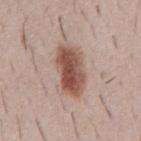Q: Was a biopsy performed?
A: total-body-photography surveillance lesion; no biopsy
Q: What is the imaging modality?
A: ~15 mm crop, total-body skin-cancer survey
Q: Who is the patient?
A: male, about 50 years old
Q: Illumination type?
A: white-light illumination
Q: What is the lesion's diameter?
A: ~6 mm (longest diameter)
Q: Automated lesion metrics?
A: a lesion area of about 15 mm², a shape eccentricity near 0.85, and two-axis asymmetry of about 0.2; an automated nevus-likeness rating near 100 out of 100
Q: Where on the body is the lesion?
A: the chest